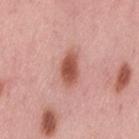Acquisition and patient details: A female subject, about 50 years old. This is a white-light tile. Approximately 4 mm at its widest. The lesion is on the lower back. A 15 mm close-up tile from a total-body photography series done for melanoma screening.